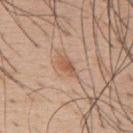{
  "biopsy_status": "not biopsied; imaged during a skin examination",
  "patient": {
    "sex": "male",
    "age_approx": 55
  },
  "image": {
    "source": "total-body photography crop",
    "field_of_view_mm": 15
  },
  "site": "upper back"
}